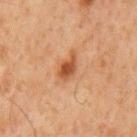| key | value |
|---|---|
| follow-up | total-body-photography surveillance lesion; no biopsy |
| patient | male, in their 60s |
| diameter | about 3.5 mm |
| acquisition | 15 mm crop, total-body photography |
| location | the back |